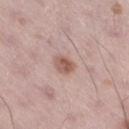workup: imaged on a skin check; not biopsied | image: ~15 mm tile from a whole-body skin photo | illumination: white-light | patient: male, aged approximately 55 | size: about 2.5 mm | location: the right thigh.